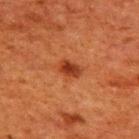biopsy_status: not biopsied; imaged during a skin examination
site: upper back
patient:
  sex: male
  age_approx: 60
image:
  source: total-body photography crop
  field_of_view_mm: 15
automated_metrics:
  eccentricity: 0.75
  shape_asymmetry: 0.2
  cielab_L: 31
  cielab_a: 26
  cielab_b: 32
  vs_skin_darker_L: 10.0
  vs_skin_contrast_norm: 9.0
  border_irregularity_0_10: 1.5
  color_variation_0_10: 2.5